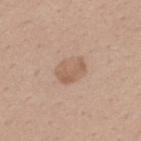{
  "patient": {
    "sex": "male",
    "age_approx": 40
  },
  "lighting": "white-light",
  "automated_metrics": {
    "cielab_L": 59,
    "cielab_a": 18,
    "cielab_b": 29,
    "vs_skin_darker_L": 8.0,
    "vs_skin_contrast_norm": 5.5,
    "border_irregularity_0_10": 1.5,
    "peripheral_color_asymmetry": 1.0
  },
  "image": {
    "source": "total-body photography crop",
    "field_of_view_mm": 15
  },
  "site": "upper back",
  "lesion_size": {
    "long_diameter_mm_approx": 3.5
  }
}